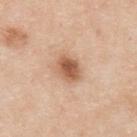{
  "biopsy_status": "not biopsied; imaged during a skin examination",
  "site": "upper back",
  "image": {
    "source": "total-body photography crop",
    "field_of_view_mm": 15
  },
  "lesion_size": {
    "long_diameter_mm_approx": 3.0
  },
  "patient": {
    "sex": "male",
    "age_approx": 35
  },
  "lighting": "white-light"
}